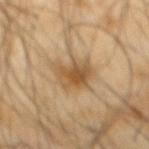The lesion was photographed on a routine skin check and not biopsied; there is no pathology result.
The lesion-visualizer software estimated a lesion area of about 10 mm² and an outline eccentricity of about 0.4 (0 = round, 1 = elongated). It also reported border irregularity of about 3.5 on a 0–10 scale and a peripheral color-asymmetry measure near 1.5. It also reported a nevus-likeness score of about 85/100 and a detector confidence of about 100 out of 100 that the crop contains a lesion.
About 4 mm across.
A male subject, in their mid- to late 60s.
Located on the back.
A region of skin cropped from a whole-body photographic capture, roughly 15 mm wide.